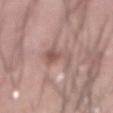Image and clinical context: A region of skin cropped from a whole-body photographic capture, roughly 15 mm wide. The lesion is on the abdomen. The tile uses white-light illumination. Approximately 5 mm at its widest. A male subject aged 58–62.